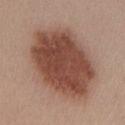Assessment: The lesion was photographed on a routine skin check and not biopsied; there is no pathology result. Acquisition and patient details: The subject is a female approximately 45 years of age. Automated tile analysis of the lesion measured an eccentricity of roughly 0.75 and a shape-asymmetry score of about 0.1 (0 = symmetric). The software also gave a mean CIELAB color near L≈46 a*≈22 b*≈27 and roughly 15 lightness units darker than nearby skin. The lesion is located on the front of the torso. A 15 mm close-up tile from a total-body photography series done for melanoma screening.